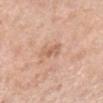The lesion was photographed on a routine skin check and not biopsied; there is no pathology result.
A roughly 15 mm field-of-view crop from a total-body skin photograph.
The lesion is located on the right lower leg.
Measured at roughly 3 mm in maximum diameter.
Captured under white-light illumination.
A female patient roughly 70 years of age.